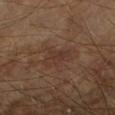A male patient aged 68 to 72. Automated image analysis of the tile measured an area of roughly 6 mm², an eccentricity of roughly 0.8, and two-axis asymmetry of about 0.3. And it measured an automated nevus-likeness rating near 0 out of 100 and a lesion-detection confidence of about 100/100. The lesion is located on the right forearm. A 15 mm close-up tile from a total-body photography series done for melanoma screening.